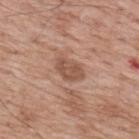| field | value |
|---|---|
| workup | imaged on a skin check; not biopsied |
| lesion size | about 3.5 mm |
| image source | ~15 mm tile from a whole-body skin photo |
| tile lighting | white-light |
| location | the upper back |
| subject | male, in their 70s |